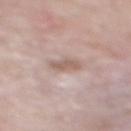No biopsy was performed on this lesion — it was imaged during a full skin examination and was not determined to be concerning. Cropped from a total-body skin-imaging series; the visible field is about 15 mm. A male subject, aged approximately 60. Located on the mid back. The lesion-visualizer software estimated an outline eccentricity of about 0.8 (0 = round, 1 = elongated) and two-axis asymmetry of about 0.25. The analysis additionally found a border-irregularity rating of about 2.5/10, a within-lesion color-variation index near 1.5/10, and radial color variation of about 0.5. The lesion's longest dimension is about 3 mm.